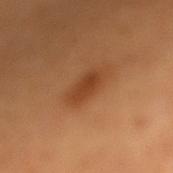biopsy status: no biopsy performed (imaged during a skin exam) | acquisition: total-body-photography crop, ~15 mm field of view | image-analysis metrics: an eccentricity of roughly 0.8 and a shape-asymmetry score of about 0.2 (0 = symmetric); an average lesion color of about L≈42 a*≈24 b*≈36 (CIELAB), a lesion–skin lightness drop of about 9, and a lesion-to-skin contrast of about 7 (normalized; higher = more distinct); border irregularity of about 2.5 on a 0–10 scale, a within-lesion color-variation index near 2.5/10, and radial color variation of about 0.5; a classifier nevus-likeness of about 90/100 and lesion-presence confidence of about 100/100 | patient: female, roughly 45 years of age | illumination: cross-polarized | lesion diameter: about 4 mm | body site: the leg.